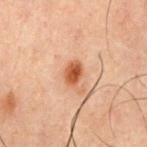Clinical impression:
This lesion was catalogued during total-body skin photography and was not selected for biopsy.
Context:
Located on the chest. The tile uses cross-polarized illumination. A region of skin cropped from a whole-body photographic capture, roughly 15 mm wide. About 3 mm across. The subject is a male in their 60s. Automated tile analysis of the lesion measured a footprint of about 5 mm², a shape eccentricity near 0.7, and a symmetry-axis asymmetry near 0.2. It also reported a lesion–skin lightness drop of about 11 and a lesion-to-skin contrast of about 9.5 (normalized; higher = more distinct). And it measured an automated nevus-likeness rating near 95 out of 100 and a lesion-detection confidence of about 100/100.